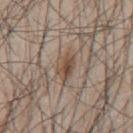The lesion was photographed on a routine skin check and not biopsied; there is no pathology result. The recorded lesion diameter is about 4 mm. Automated tile analysis of the lesion measured a mean CIELAB color near L≈49 a*≈14 b*≈26, roughly 8 lightness units darker than nearby skin, and a normalized lesion–skin contrast near 7. The software also gave a border-irregularity index near 2.5/10, internal color variation of about 6 on a 0–10 scale, and radial color variation of about 2. On the mid back. Cropped from a whole-body photographic skin survey; the tile spans about 15 mm. This is a white-light tile. A male subject, aged around 45.